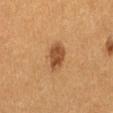Assessment:
Imaged during a routine full-body skin examination; the lesion was not biopsied and no histopathology is available.
Background:
From the right thigh. Automated tile analysis of the lesion measured an area of roughly 7 mm², a shape eccentricity near 0.7, and a shape-asymmetry score of about 0.15 (0 = symmetric). The software also gave about 10 CIELAB-L* units darker than the surrounding skin and a normalized lesion–skin contrast near 8.5. Longest diameter approximately 3.5 mm. A region of skin cropped from a whole-body photographic capture, roughly 15 mm wide. This is a cross-polarized tile. The patient is a female roughly 40 years of age.